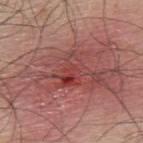A 15 mm close-up tile from a total-body photography series done for melanoma screening. The lesion is located on the upper back. A male patient, approximately 45 years of age. This is a white-light tile. On biopsy, histopathology showed a seborrheic keratosis.